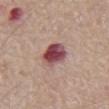workup = catalogued during a skin exam; not biopsied | location = the abdomen | image source = total-body-photography crop, ~15 mm field of view | subject = male, aged around 65.